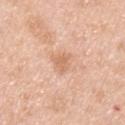Assessment:
Part of a total-body skin-imaging series; this lesion was reviewed on a skin check and was not flagged for biopsy.
Background:
Located on the chest. A male subject approximately 45 years of age. A close-up tile cropped from a whole-body skin photograph, about 15 mm across. Captured under white-light illumination. Approximately 2.5 mm at its widest.